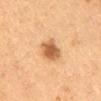| key | value |
|---|---|
| lesion diameter | ≈4.5 mm |
| patient | female, aged 38–42 |
| automated lesion analysis | a color-variation rating of about 4/10 and peripheral color asymmetry of about 1 |
| tile lighting | cross-polarized illumination |
| body site | the back |
| acquisition | 15 mm crop, total-body photography |